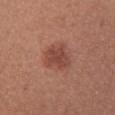| key | value |
|---|---|
| automated lesion analysis | a footprint of about 9.5 mm², an outline eccentricity of about 0.7 (0 = round, 1 = elongated), and a shape-asymmetry score of about 0.2 (0 = symmetric); a nevus-likeness score of about 80/100 |
| diameter | ~4 mm (longest diameter) |
| location | the chest |
| image | ~15 mm crop, total-body skin-cancer survey |
| lighting | white-light |
| subject | female, aged 23–27 |